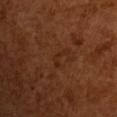The total-body-photography lesion software estimated a footprint of about 2 mm², an outline eccentricity of about 0.85 (0 = round, 1 = elongated), and a symmetry-axis asymmetry near 0.7. And it measured an average lesion color of about L≈25 a*≈21 b*≈28 (CIELAB). The lesion's longest dimension is about 2.5 mm. A lesion tile, about 15 mm wide, cut from a 3D total-body photograph. A male patient in their mid-60s. This is a cross-polarized tile.